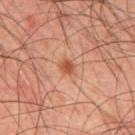  automated_metrics:
    area_mm2_approx: 3.0
    eccentricity: 0.6
    shape_asymmetry: 0.15
    cielab_L: 51
    cielab_a: 26
    cielab_b: 34
    vs_skin_darker_L: 10.0
    vs_skin_contrast_norm: 8.0
  image:
    source: total-body photography crop
    field_of_view_mm: 15
  site: mid back
  patient:
    sex: male
    age_approx: 45
  lighting: cross-polarized
  lesion_size:
    long_diameter_mm_approx: 2.0